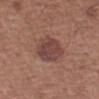diameter: ≈4.5 mm | image-analysis metrics: a mean CIELAB color near L≈43 a*≈21 b*≈22, roughly 9 lightness units darker than nearby skin, and a normalized lesion–skin contrast near 8; an automated nevus-likeness rating near 25 out of 100 | location: the left forearm | subject: male, roughly 55 years of age | imaging modality: ~15 mm crop, total-body skin-cancer survey | lighting: white-light.